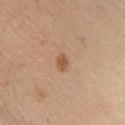Context:
Measured at roughly 2 mm in maximum diameter. A male subject, in their 50s. From the right lower leg. Captured under cross-polarized illumination. The lesion-visualizer software estimated an average lesion color of about L≈50 a*≈19 b*≈31 (CIELAB) and about 9 CIELAB-L* units darker than the surrounding skin. The analysis additionally found internal color variation of about 0.5 on a 0–10 scale and radial color variation of about 0. And it measured a classifier nevus-likeness of about 85/100 and a lesion-detection confidence of about 100/100. Cropped from a whole-body photographic skin survey; the tile spans about 15 mm.